The lesion's longest dimension is about 8.5 mm, located on the head or neck, Automated tile analysis of the lesion measured a footprint of about 25 mm² and two-axis asymmetry of about 0.3. It also reported a border-irregularity rating of about 4.5/10 and internal color variation of about 5.5 on a 0–10 scale, imaged with white-light lighting, cropped from a total-body skin-imaging series; the visible field is about 15 mm, a female patient aged approximately 40:
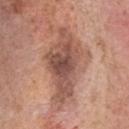On excision, pathology confirmed an atypical melanocytic neoplasm — an indeterminate (borderline) lesion.